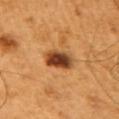No biopsy was performed on this lesion — it was imaged during a full skin examination and was not determined to be concerning.
This is a cross-polarized tile.
The lesion is on the left upper arm.
A roughly 15 mm field-of-view crop from a total-body skin photograph.
The lesion's longest dimension is about 4 mm.
A male patient roughly 60 years of age.